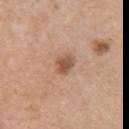The lesion is located on the right upper arm. The recorded lesion diameter is about 3 mm. An algorithmic analysis of the crop reported a shape eccentricity near 0.6. It also reported a border-irregularity index near 2/10, internal color variation of about 3.5 on a 0–10 scale, and radial color variation of about 1. A female patient, aged around 60. This image is a 15 mm lesion crop taken from a total-body photograph.